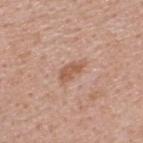| field | value |
|---|---|
| workup | total-body-photography surveillance lesion; no biopsy |
| acquisition | ~15 mm tile from a whole-body skin photo |
| location | the upper back |
| subject | male, aged approximately 40 |
| TBP lesion metrics | a nevus-likeness score of about 0/100 and lesion-presence confidence of about 100/100 |
| tile lighting | white-light illumination |